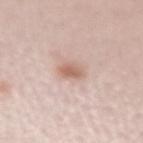Impression: Part of a total-body skin-imaging series; this lesion was reviewed on a skin check and was not flagged for biopsy. Acquisition and patient details: A lesion tile, about 15 mm wide, cut from a 3D total-body photograph. The patient is a female roughly 45 years of age. Captured under white-light illumination. The lesion is on the arm. The recorded lesion diameter is about 2.5 mm.